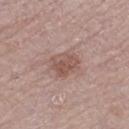Captured during whole-body skin photography for melanoma surveillance; the lesion was not biopsied. The lesion is on the left thigh. A 15 mm crop from a total-body photograph taken for skin-cancer surveillance. The subject is a male roughly 75 years of age.